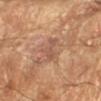This lesion was catalogued during total-body skin photography and was not selected for biopsy.
From the arm.
Captured under cross-polarized illumination.
This image is a 15 mm lesion crop taken from a total-body photograph.
The patient is a male aged approximately 65.